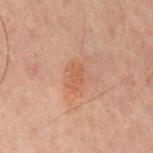biopsy status — no biopsy performed (imaged during a skin exam) | image-analysis metrics — a footprint of about 5.5 mm², a shape eccentricity near 0.75, and two-axis asymmetry of about 0.35; an average lesion color of about L≈44 a*≈19 b*≈27 (CIELAB), roughly 5 lightness units darker than nearby skin, and a lesion-to-skin contrast of about 5.5 (normalized; higher = more distinct); a border-irregularity rating of about 3.5/10 and radial color variation of about 0.5; an automated nevus-likeness rating near 15 out of 100 and lesion-presence confidence of about 100/100 | anatomic site — the chest | lesion diameter — about 3.5 mm | illumination — cross-polarized | acquisition — 15 mm crop, total-body photography | subject — male, aged 58–62.